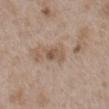biopsy_status: not biopsied; imaged during a skin examination
image:
  source: total-body photography crop
  field_of_view_mm: 15
site: mid back
patient:
  sex: male
  age_approx: 50